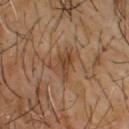Q: Was a biopsy performed?
A: imaged on a skin check; not biopsied
Q: Who is the patient?
A: male, about 65 years old
Q: What is the anatomic site?
A: the upper back
Q: What did automated image analysis measure?
A: a lesion area of about 5 mm² and a shape eccentricity near 0.75; an average lesion color of about L≈40 a*≈18 b*≈29 (CIELAB) and a normalized border contrast of about 6.5; a border-irregularity rating of about 4.5/10, a within-lesion color-variation index near 3.5/10, and radial color variation of about 1.5
Q: What is the lesion's diameter?
A: ≈3.5 mm
Q: How was this image acquired?
A: ~15 mm tile from a whole-body skin photo
Q: Illumination type?
A: cross-polarized illumination